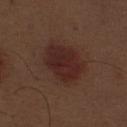The lesion is on the abdomen. The subject is a male in their 70s. A region of skin cropped from a whole-body photographic capture, roughly 15 mm wide. The lesion's longest dimension is about 5.5 mm. This is a white-light tile.